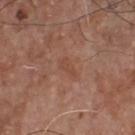Clinical impression:
The lesion was photographed on a routine skin check and not biopsied; there is no pathology result.
Image and clinical context:
On the front of the torso. A region of skin cropped from a whole-body photographic capture, roughly 15 mm wide. A male subject aged around 75. The tile uses white-light illumination. An algorithmic analysis of the crop reported a border-irregularity index near 4/10 and radial color variation of about 0.5. Longest diameter approximately 3 mm.